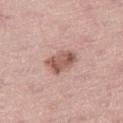Part of a total-body skin-imaging series; this lesion was reviewed on a skin check and was not flagged for biopsy.
Approximately 3.5 mm at its widest.
Imaged with white-light lighting.
The patient is a male approximately 60 years of age.
A 15 mm close-up tile from a total-body photography series done for melanoma screening.
The lesion is located on the leg.